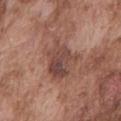No biopsy was performed on this lesion — it was imaged during a full skin examination and was not determined to be concerning. A 15 mm crop from a total-body photograph taken for skin-cancer surveillance. A male subject roughly 75 years of age. Imaged with white-light lighting. The lesion is on the back. The lesion's longest dimension is about 5 mm.